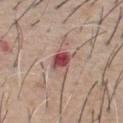workup: imaged on a skin check; not biopsied | subject: male, approximately 60 years of age | acquisition: ~15 mm tile from a whole-body skin photo | image-analysis metrics: an area of roughly 6.5 mm², an outline eccentricity of about 0.7 (0 = round, 1 = elongated), and a shape-asymmetry score of about 0.25 (0 = symmetric); roughly 13 lightness units darker than nearby skin and a lesion-to-skin contrast of about 9.5 (normalized; higher = more distinct); a border-irregularity rating of about 2.5/10; a classifier nevus-likeness of about 5/100 and a detector confidence of about 100 out of 100 that the crop contains a lesion | lesion size: ≈3.5 mm | illumination: white-light illumination | anatomic site: the chest.